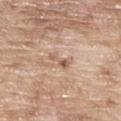Imaged during a routine full-body skin examination; the lesion was not biopsied and no histopathology is available. A male subject aged around 65. A 15 mm close-up extracted from a 3D total-body photography capture. The lesion is located on the upper back. Imaged with white-light lighting.